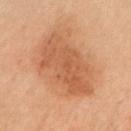follow-up=total-body-photography surveillance lesion; no biopsy | lesion diameter=about 9 mm | patient=female, approximately 65 years of age | location=the right forearm | image=~15 mm crop, total-body skin-cancer survey | automated lesion analysis=border irregularity of about 3.5 on a 0–10 scale and internal color variation of about 3.5 on a 0–10 scale.